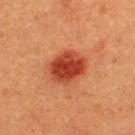<lesion>
<patient>
  <sex>male</sex>
  <age_approx>40</age_approx>
</patient>
<image>
  <source>total-body photography crop</source>
  <field_of_view_mm>15</field_of_view_mm>
</image>
<site>upper back</site>
<lighting>cross-polarized</lighting>
<lesion_size>
  <long_diameter_mm_approx>4.5</long_diameter_mm_approx>
</lesion_size>
</lesion>